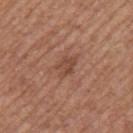Recorded during total-body skin imaging; not selected for excision or biopsy. A close-up tile cropped from a whole-body skin photograph, about 15 mm across. Approximately 3 mm at its widest. The subject is a female approximately 65 years of age. Located on the arm.